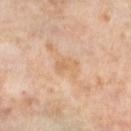This lesion was catalogued during total-body skin photography and was not selected for biopsy. The lesion is on the left lower leg. Captured under cross-polarized illumination. This image is a 15 mm lesion crop taken from a total-body photograph. Approximately 3 mm at its widest. A female subject, about 55 years old.